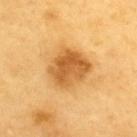  biopsy_status: not biopsied; imaged during a skin examination
  automated_metrics:
    area_mm2_approx: 16.0
    eccentricity: 0.5
    shape_asymmetry: 0.15
    vs_skin_darker_L: 14.0
    border_irregularity_0_10: 1.5
    color_variation_0_10: 5.0
    peripheral_color_asymmetry: 2.0
    nevus_likeness_0_100: 65
  patient:
    sex: male
    age_approx: 60
  site: upper back
  image:
    source: total-body photography crop
    field_of_view_mm: 15
  lighting: cross-polarized